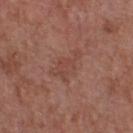– patient: male, aged 53–57
– diameter: about 4 mm
– location: the chest
– acquisition: total-body-photography crop, ~15 mm field of view